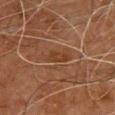biopsy status = catalogued during a skin exam; not biopsied
anatomic site = the chest
image source = ~15 mm crop, total-body skin-cancer survey
lesion size = ~2.5 mm (longest diameter)
patient = male, about 65 years old
tile lighting = cross-polarized
image-analysis metrics = a footprint of about 3 mm² and two-axis asymmetry of about 0.3; about 6 CIELAB-L* units darker than the surrounding skin and a normalized border contrast of about 6.5; a nevus-likeness score of about 0/100 and a lesion-detection confidence of about 90/100